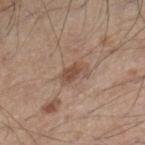{
  "biopsy_status": "not biopsied; imaged during a skin examination",
  "image": {
    "source": "total-body photography crop",
    "field_of_view_mm": 15
  },
  "patient": {
    "sex": "male",
    "age_approx": 45
  },
  "site": "right lower leg",
  "lighting": "white-light",
  "automated_metrics": {
    "vs_skin_darker_L": 10.0,
    "vs_skin_contrast_norm": 7.5
  },
  "lesion_size": {
    "long_diameter_mm_approx": 3.5
  }
}